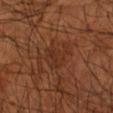A lesion tile, about 15 mm wide, cut from a 3D total-body photograph.
The lesion is located on the left forearm.
Automated tile analysis of the lesion measured a border-irregularity index near 6/10 and radial color variation of about 1. The software also gave a nevus-likeness score of about 0/100 and a lesion-detection confidence of about 100/100.
About 4 mm across.
The patient is a male about 65 years old.
Captured under cross-polarized illumination.